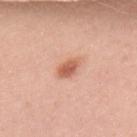Q: Was a biopsy performed?
A: total-body-photography surveillance lesion; no biopsy
Q: What are the patient's age and sex?
A: female, roughly 30 years of age
Q: What is the imaging modality?
A: total-body-photography crop, ~15 mm field of view
Q: What is the anatomic site?
A: the right upper arm